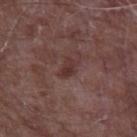Approximately 2.5 mm at its widest.
The patient is a male in their mid- to late 60s.
Captured under white-light illumination.
A 15 mm close-up tile from a total-body photography series done for melanoma screening.
The lesion is on the left upper arm.
Automated image analysis of the tile measured a border-irregularity index near 3.5/10 and a within-lesion color-variation index near 2.5/10. The analysis additionally found a nevus-likeness score of about 0/100 and lesion-presence confidence of about 100/100.